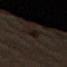A 15 mm close-up extracted from a 3D total-body photography capture.
The lesion is located on the left thigh.
The total-body-photography lesion software estimated a border-irregularity rating of about 3.5/10 and internal color variation of about 2 on a 0–10 scale.
A male subject in their mid- to late 80s or older.
Imaged with cross-polarized lighting.
The lesion's longest dimension is about 3.5 mm.